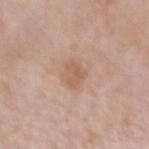Case summary:
- follow-up · catalogued during a skin exam; not biopsied
- lesion diameter · ~3 mm (longest diameter)
- image · ~15 mm tile from a whole-body skin photo
- patient · female, in their mid-60s
- TBP lesion metrics · a lesion color around L≈59 a*≈20 b*≈30 in CIELAB, roughly 8 lightness units darker than nearby skin, and a normalized border contrast of about 5.5; a border-irregularity rating of about 2/10, internal color variation of about 2.5 on a 0–10 scale, and a peripheral color-asymmetry measure near 1; an automated nevus-likeness rating near 0 out of 100 and a lesion-detection confidence of about 100/100
- site · the right forearm
- illumination · white-light